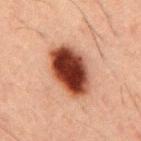acquisition=15 mm crop, total-body photography | patient=male, roughly 50 years of age | tile lighting=cross-polarized | anatomic site=the mid back.